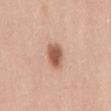{"biopsy_status": "not biopsied; imaged during a skin examination", "site": "lower back", "lesion_size": {"long_diameter_mm_approx": 3.0}, "patient": {"sex": "female", "age_approx": 30}, "image": {"source": "total-body photography crop", "field_of_view_mm": 15}, "lighting": "white-light"}